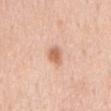notes = catalogued during a skin exam; not biopsied
TBP lesion metrics = an area of roughly 4 mm², a shape eccentricity near 0.65, and a symmetry-axis asymmetry near 0.3; border irregularity of about 2.5 on a 0–10 scale and a within-lesion color-variation index near 3/10
illumination = white-light illumination
lesion size = ~2.5 mm (longest diameter)
image = ~15 mm crop, total-body skin-cancer survey
location = the mid back
patient = male, approximately 60 years of age